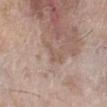  biopsy_status: not biopsied; imaged during a skin examination
  site: left lower leg
  automated_metrics:
    area_mm2_approx: 3.0
    eccentricity: 0.85
    shape_asymmetry: 0.6
    border_irregularity_0_10: 6.5
    color_variation_0_10: 0.5
    peripheral_color_asymmetry: 0.0
  lighting: white-light
  patient:
    sex: female
    age_approx: 75
  image:
    source: total-body photography crop
    field_of_view_mm: 15
  lesion_size:
    long_diameter_mm_approx: 3.0